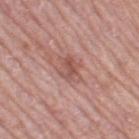The lesion was photographed on a routine skin check and not biopsied; there is no pathology result. Imaged with white-light lighting. The patient is a female roughly 65 years of age. From the left thigh. A region of skin cropped from a whole-body photographic capture, roughly 15 mm wide.